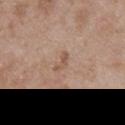{
  "biopsy_status": "not biopsied; imaged during a skin examination",
  "image": {
    "source": "total-body photography crop",
    "field_of_view_mm": 15
  },
  "site": "right upper arm",
  "patient": {
    "sex": "male",
    "age_approx": 50
  },
  "lesion_size": {
    "long_diameter_mm_approx": 2.5
  },
  "lighting": "white-light"
}